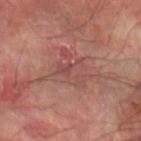  biopsy_status: not biopsied; imaged during a skin examination
  site: arm
  patient:
    sex: male
    age_approx: 65
  image:
    source: total-body photography crop
    field_of_view_mm: 15
  lighting: cross-polarized
  lesion_size:
    long_diameter_mm_approx: 7.0